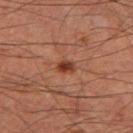This lesion was catalogued during total-body skin photography and was not selected for biopsy. From the left thigh. A male subject, about 60 years old. Cropped from a whole-body photographic skin survey; the tile spans about 15 mm.